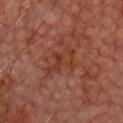Part of a total-body skin-imaging series; this lesion was reviewed on a skin check and was not flagged for biopsy.
On the chest.
The tile uses cross-polarized illumination.
Approximately 4.5 mm at its widest.
The patient is a male in their mid- to late 70s.
A lesion tile, about 15 mm wide, cut from a 3D total-body photograph.